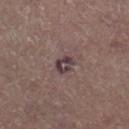<case>
  <biopsy_status>not biopsied; imaged during a skin examination</biopsy_status>
  <image>
    <source>total-body photography crop</source>
    <field_of_view_mm>15</field_of_view_mm>
  </image>
  <site>right lower leg</site>
  <lesion_size>
    <long_diameter_mm_approx>2.5</long_diameter_mm_approx>
  </lesion_size>
  <patient>
    <sex>male</sex>
    <age_approx>65</age_approx>
  </patient>
</case>